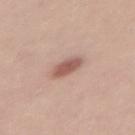{
  "biopsy_status": "not biopsied; imaged during a skin examination",
  "lighting": "white-light",
  "image": {
    "source": "total-body photography crop",
    "field_of_view_mm": 15
  },
  "lesion_size": {
    "long_diameter_mm_approx": 3.0
  },
  "automated_metrics": {
    "area_mm2_approx": 5.5,
    "shape_asymmetry": 0.2,
    "cielab_L": 56,
    "cielab_a": 22,
    "cielab_b": 25,
    "vs_skin_darker_L": 13.0,
    "vs_skin_contrast_norm": 8.0,
    "nevus_likeness_0_100": 95
  },
  "patient": {
    "sex": "female",
    "age_approx": 35
  },
  "site": "lower back"
}